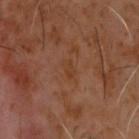Captured during whole-body skin photography for melanoma surveillance; the lesion was not biopsied. Located on the head or neck. A male patient roughly 60 years of age. A 15 mm close-up extracted from a 3D total-body photography capture.